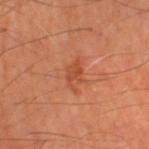Part of a total-body skin-imaging series; this lesion was reviewed on a skin check and was not flagged for biopsy. From the left thigh. The recorded lesion diameter is about 3.5 mm. Automated image analysis of the tile measured an area of roughly 4 mm², an eccentricity of roughly 0.85, and two-axis asymmetry of about 0.4. And it measured about 8 CIELAB-L* units darker than the surrounding skin. The analysis additionally found a classifier nevus-likeness of about 0/100 and lesion-presence confidence of about 100/100. A male patient aged approximately 70. The tile uses cross-polarized illumination. A roughly 15 mm field-of-view crop from a total-body skin photograph.